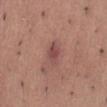<lesion>
  <biopsy_status>not biopsied; imaged during a skin examination</biopsy_status>
  <automated_metrics>
    <vs_skin_darker_L>9.0</vs_skin_darker_L>
  </automated_metrics>
  <site>abdomen</site>
  <lesion_size>
    <long_diameter_mm_approx>3.0</long_diameter_mm_approx>
  </lesion_size>
  <lighting>white-light</lighting>
  <image>
    <source>total-body photography crop</source>
    <field_of_view_mm>15</field_of_view_mm>
  </image>
  <patient>
    <sex>male</sex>
    <age_approx>45</age_approx>
  </patient>
</lesion>